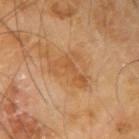Recorded during total-body skin imaging; not selected for excision or biopsy. A male subject aged around 65. A 15 mm close-up extracted from a 3D total-body photography capture. An algorithmic analysis of the crop reported a footprint of about 9.5 mm², an eccentricity of roughly 0.75, and a shape-asymmetry score of about 0.65 (0 = symmetric). And it measured an average lesion color of about L≈55 a*≈22 b*≈40 (CIELAB), a lesion–skin lightness drop of about 7, and a normalized lesion–skin contrast near 5.5. On the right upper arm. The tile uses cross-polarized illumination.